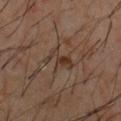Imaged during a routine full-body skin examination; the lesion was not biopsied and no histopathology is available.
A close-up tile cropped from a whole-body skin photograph, about 15 mm across.
The lesion is on the chest.
About 3.5 mm across.
A male patient, aged 58–62.